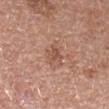The lesion was photographed on a routine skin check and not biopsied; there is no pathology result. The patient is a female aged around 45. Imaged with white-light lighting. The lesion is on the right forearm. A region of skin cropped from a whole-body photographic capture, roughly 15 mm wide.